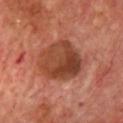Assessment:
The lesion was tiled from a total-body skin photograph and was not biopsied.
Image and clinical context:
The lesion is on the chest. The total-body-photography lesion software estimated a lesion color around L≈42 a*≈26 b*≈31 in CIELAB, roughly 11 lightness units darker than nearby skin, and a normalized lesion–skin contrast near 8.5. A close-up tile cropped from a whole-body skin photograph, about 15 mm across. A male subject aged around 65.